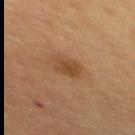The lesion was tiled from a total-body skin photograph and was not biopsied.
The subject is a male aged 58 to 62.
The recorded lesion diameter is about 3 mm.
An algorithmic analysis of the crop reported an average lesion color of about L≈40 a*≈18 b*≈31 (CIELAB), roughly 8 lightness units darker than nearby skin, and a lesion-to-skin contrast of about 7 (normalized; higher = more distinct). The analysis additionally found a lesion-detection confidence of about 100/100.
This is a cross-polarized tile.
A 15 mm close-up tile from a total-body photography series done for melanoma screening.
From the back.